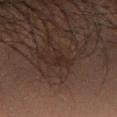<lesion>
<biopsy_status>not biopsied; imaged during a skin examination</biopsy_status>
<patient>
  <sex>male</sex>
  <age_approx>70</age_approx>
</patient>
<lesion_size>
  <long_diameter_mm_approx>2.5</long_diameter_mm_approx>
</lesion_size>
<image>
  <source>total-body photography crop</source>
  <field_of_view_mm>15</field_of_view_mm>
</image>
<site>arm</site>
<lighting>cross-polarized</lighting>
<automated_metrics>
  <area_mm2_approx>2.5</area_mm2_approx>
  <eccentricity>0.9</eccentricity>
  <shape_asymmetry>0.5</shape_asymmetry>
</automated_metrics>
</lesion>